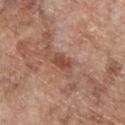Recorded during total-body skin imaging; not selected for excision or biopsy. A 15 mm close-up extracted from a 3D total-body photography capture. The patient is a male aged 68–72. This is a white-light tile. Longest diameter approximately 3 mm. Located on the upper back.